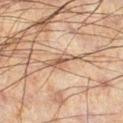An algorithmic analysis of the crop reported an area of roughly 3 mm², an outline eccentricity of about 0.95 (0 = round, 1 = elongated), and a symmetry-axis asymmetry near 0.35. The software also gave a lesion–skin lightness drop of about 10 and a normalized border contrast of about 8. The software also gave a border-irregularity index near 4/10, internal color variation of about 0 on a 0–10 scale, and peripheral color asymmetry of about 0.
On the left leg.
A male patient, aged 58–62.
Approximately 3 mm at its widest.
A 15 mm close-up extracted from a 3D total-body photography capture.